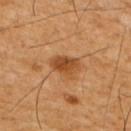Captured during whole-body skin photography for melanoma surveillance; the lesion was not biopsied. The subject is a male aged 58 to 62. A close-up tile cropped from a whole-body skin photograph, about 15 mm across. The lesion's longest dimension is about 3.5 mm. The lesion is on the arm. The total-body-photography lesion software estimated an outline eccentricity of about 0.75 (0 = round, 1 = elongated) and two-axis asymmetry of about 0.25. The analysis additionally found a lesion color around L≈43 a*≈22 b*≈36 in CIELAB, a lesion–skin lightness drop of about 10, and a lesion-to-skin contrast of about 8 (normalized; higher = more distinct). The software also gave a within-lesion color-variation index near 4/10. And it measured a nevus-likeness score of about 75/100 and a lesion-detection confidence of about 100/100.